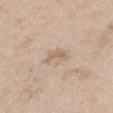Imaged during a routine full-body skin examination; the lesion was not biopsied and no histopathology is available. On the front of the torso. An algorithmic analysis of the crop reported a footprint of about 4 mm², a shape eccentricity near 0.75, and a shape-asymmetry score of about 0.45 (0 = symmetric). And it measured an average lesion color of about L≈63 a*≈14 b*≈30 (CIELAB), roughly 8 lightness units darker than nearby skin, and a normalized border contrast of about 5.5. And it measured border irregularity of about 5 on a 0–10 scale and a within-lesion color-variation index near 1/10. A female patient, aged 28 to 32. Cropped from a total-body skin-imaging series; the visible field is about 15 mm. The tile uses white-light illumination.